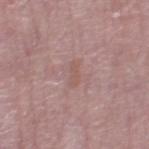{"biopsy_status": "not biopsied; imaged during a skin examination", "patient": {"sex": "female", "age_approx": 65}, "image": {"source": "total-body photography crop", "field_of_view_mm": 15}, "lesion_size": {"long_diameter_mm_approx": 2.5}, "site": "right thigh", "lighting": "white-light"}